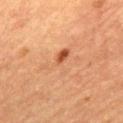Clinical impression: Imaged during a routine full-body skin examination; the lesion was not biopsied and no histopathology is available. Clinical summary: About 5 mm across. A 15 mm close-up tile from a total-body photography series done for melanoma screening. A female subject, aged 68–72. The lesion-visualizer software estimated a lesion area of about 9 mm², a shape eccentricity near 0.85, and a shape-asymmetry score of about 0.5 (0 = symmetric). From the mid back.